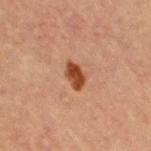{
  "site": "right forearm",
  "lighting": "cross-polarized",
  "image": {
    "source": "total-body photography crop",
    "field_of_view_mm": 15
  },
  "patient": {
    "sex": "female",
    "age_approx": 70
  },
  "lesion_size": {
    "long_diameter_mm_approx": 3.0
  }
}